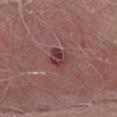No biopsy was performed on this lesion — it was imaged during a full skin examination and was not determined to be concerning. The recorded lesion diameter is about 2.5 mm. The lesion-visualizer software estimated an outline eccentricity of about 0.5 (0 = round, 1 = elongated). And it measured a border-irregularity index near 2/10 and a within-lesion color-variation index near 7.5/10. Imaged with white-light lighting. Located on the abdomen. A lesion tile, about 15 mm wide, cut from a 3D total-body photograph. A male subject about 75 years old.